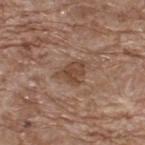A male patient in their 70s. This image is a 15 mm lesion crop taken from a total-body photograph. On the upper back.